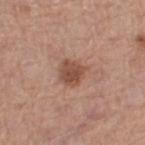Impression: No biopsy was performed on this lesion — it was imaged during a full skin examination and was not determined to be concerning. Context: A female subject, in their mid-50s. On the left thigh. A close-up tile cropped from a whole-body skin photograph, about 15 mm across.